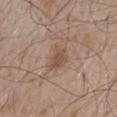Assessment: This lesion was catalogued during total-body skin photography and was not selected for biopsy. Clinical summary: Longest diameter approximately 3.5 mm. Located on the mid back. Cropped from a total-body skin-imaging series; the visible field is about 15 mm. A male subject in their mid- to late 50s.